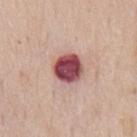The lesion was photographed on a routine skin check and not biopsied; there is no pathology result. On the chest. A close-up tile cropped from a whole-body skin photograph, about 15 mm across. The recorded lesion diameter is about 3.5 mm. The subject is a male approximately 60 years of age.